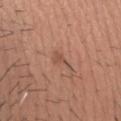The lesion was tiled from a total-body skin photograph and was not biopsied.
The lesion's longest dimension is about 2.5 mm.
The total-body-photography lesion software estimated a footprint of about 3 mm² and two-axis asymmetry of about 0.5. It also reported a mean CIELAB color near L≈51 a*≈22 b*≈29 and about 7 CIELAB-L* units darker than the surrounding skin. The analysis additionally found a within-lesion color-variation index near 1/10. It also reported a nevus-likeness score of about 0/100 and a lesion-detection confidence of about 100/100.
A region of skin cropped from a whole-body photographic capture, roughly 15 mm wide.
A male patient, aged 58 to 62.
The tile uses white-light illumination.
Located on the head or neck.